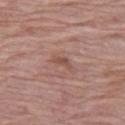Image and clinical context:
This is a white-light tile. A female patient, aged 63 to 67. A region of skin cropped from a whole-body photographic capture, roughly 15 mm wide. From the left thigh. Approximately 2.5 mm at its widest.